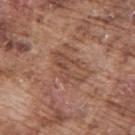Assessment:
Part of a total-body skin-imaging series; this lesion was reviewed on a skin check and was not flagged for biopsy.
Acquisition and patient details:
The tile uses white-light illumination. A male subject, in their mid-70s. Measured at roughly 5 mm in maximum diameter. A roughly 15 mm field-of-view crop from a total-body skin photograph. The lesion is on the upper back.